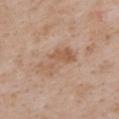Assessment:
Captured during whole-body skin photography for melanoma surveillance; the lesion was not biopsied.
Clinical summary:
The subject is a female aged around 30. A 15 mm crop from a total-body photograph taken for skin-cancer surveillance. The tile uses white-light illumination. Automated image analysis of the tile measured a border-irregularity rating of about 7.5/10, internal color variation of about 2 on a 0–10 scale, and radial color variation of about 0.5. The software also gave an automated nevus-likeness rating near 0 out of 100. The recorded lesion diameter is about 5 mm. Located on the upper back.